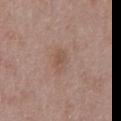biopsy status — catalogued during a skin exam; not biopsied | patient — male, aged around 50 | site — the chest | image — total-body-photography crop, ~15 mm field of view.